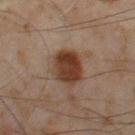Q: Is there a histopathology result?
A: no biopsy performed (imaged during a skin exam)
Q: What are the patient's age and sex?
A: male, aged approximately 45
Q: How was this image acquired?
A: total-body-photography crop, ~15 mm field of view
Q: What is the lesion's diameter?
A: ≈4.5 mm
Q: Where on the body is the lesion?
A: the left thigh